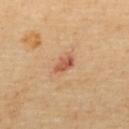A female patient in their 70s.
A roughly 15 mm field-of-view crop from a total-body skin photograph.
The lesion is located on the upper back.
This is a cross-polarized tile.
An algorithmic analysis of the crop reported border irregularity of about 2 on a 0–10 scale, a within-lesion color-variation index near 3/10, and a peripheral color-asymmetry measure near 1. And it measured a nevus-likeness score of about 15/100 and a lesion-detection confidence of about 100/100.
Measured at roughly 2.5 mm in maximum diameter.